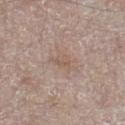The lesion was tiled from a total-body skin photograph and was not biopsied. The lesion is located on the left lower leg. The lesion's longest dimension is about 3 mm. Captured under white-light illumination. A close-up tile cropped from a whole-body skin photograph, about 15 mm across. The subject is a male roughly 65 years of age.